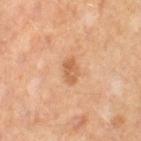Case summary:
* workup — total-body-photography surveillance lesion; no biopsy
* tile lighting — cross-polarized
* lesion size — ≈3 mm
* acquisition — 15 mm crop, total-body photography
* site — the left thigh
* patient — female, approximately 50 years of age
* TBP lesion metrics — an average lesion color of about L≈59 a*≈22 b*≈37 (CIELAB), roughly 9 lightness units darker than nearby skin, and a normalized lesion–skin contrast near 6.5; a nevus-likeness score of about 5/100